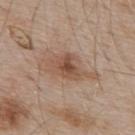The lesion was tiled from a total-body skin photograph and was not biopsied.
The subject is a male about 55 years old.
A 15 mm crop from a total-body photograph taken for skin-cancer surveillance.
From the upper back.
Longest diameter approximately 6.5 mm.
Automated image analysis of the tile measured a mean CIELAB color near L≈52 a*≈18 b*≈29, about 10 CIELAB-L* units darker than the surrounding skin, and a lesion-to-skin contrast of about 7.5 (normalized; higher = more distinct).
The tile uses white-light illumination.